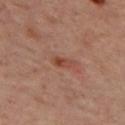{"biopsy_status": "not biopsied; imaged during a skin examination", "lighting": "cross-polarized", "lesion_size": {"long_diameter_mm_approx": 2.5}, "automated_metrics": {"area_mm2_approx": 3.0, "eccentricity": 0.85, "shape_asymmetry": 0.25, "border_irregularity_0_10": 2.5, "color_variation_0_10": 4.0, "peripheral_color_asymmetry": 1.0}, "image": {"source": "total-body photography crop", "field_of_view_mm": 15}, "patient": {"sex": "female", "age_approx": 45}, "site": "upper back"}